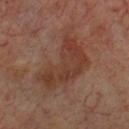Captured under cross-polarized illumination. A lesion tile, about 15 mm wide, cut from a 3D total-body photograph. A male patient, aged 68 to 72. Approximately 8 mm at its widest. Located on the chest.